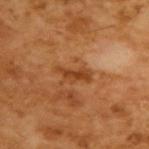Part of a total-body skin-imaging series; this lesion was reviewed on a skin check and was not flagged for biopsy.